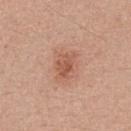{
  "biopsy_status": "not biopsied; imaged during a skin examination",
  "image": {
    "source": "total-body photography crop",
    "field_of_view_mm": 15
  },
  "lesion_size": {
    "long_diameter_mm_approx": 3.0
  },
  "automated_metrics": {
    "area_mm2_approx": 5.5,
    "shape_asymmetry": 0.25,
    "color_variation_0_10": 3.0,
    "peripheral_color_asymmetry": 1.0,
    "nevus_likeness_0_100": 45,
    "lesion_detection_confidence_0_100": 100
  },
  "lighting": "white-light",
  "patient": {
    "sex": "male",
    "age_approx": 50
  },
  "site": "chest"
}